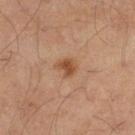Impression:
Recorded during total-body skin imaging; not selected for excision or biopsy.
Acquisition and patient details:
This is a cross-polarized tile. Approximately 2.5 mm at its widest. A region of skin cropped from a whole-body photographic capture, roughly 15 mm wide. A male subject approximately 60 years of age. From the right thigh.